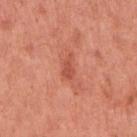notes = no biopsy performed (imaged during a skin exam) | image source = ~15 mm tile from a whole-body skin photo | patient = female, roughly 50 years of age | location = the right upper arm | tile lighting = white-light illumination | lesion size = ~2.5 mm (longest diameter).